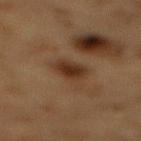Clinical impression: Part of a total-body skin-imaging series; this lesion was reviewed on a skin check and was not flagged for biopsy. Context: A male subject, in their mid- to late 80s or older. The lesion is on the back. Automated tile analysis of the lesion measured a mean CIELAB color near L≈28 a*≈16 b*≈26, roughly 9 lightness units darker than nearby skin, and a normalized border contrast of about 9.5. Measured at roughly 3.5 mm in maximum diameter. A 15 mm crop from a total-body photograph taken for skin-cancer surveillance. Imaged with cross-polarized lighting.